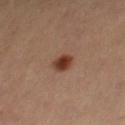Clinical impression: Imaged during a routine full-body skin examination; the lesion was not biopsied and no histopathology is available. Background: A female subject roughly 45 years of age. A close-up tile cropped from a whole-body skin photograph, about 15 mm across. From the leg. Longest diameter approximately 2.5 mm.